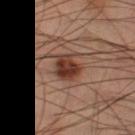{
  "biopsy_status": "not biopsied; imaged during a skin examination"
}